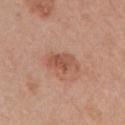Q: Was a biopsy performed?
A: catalogued during a skin exam; not biopsied
Q: Illumination type?
A: white-light illumination
Q: What did automated image analysis measure?
A: a lesion area of about 8 mm², an outline eccentricity of about 0.8 (0 = round, 1 = elongated), and a symmetry-axis asymmetry near 0.25; roughly 9 lightness units darker than nearby skin and a normalized border contrast of about 6.5; internal color variation of about 3.5 on a 0–10 scale and peripheral color asymmetry of about 1
Q: Where on the body is the lesion?
A: the left upper arm
Q: Patient demographics?
A: female, aged 53 to 57
Q: How was this image acquired?
A: ~15 mm crop, total-body skin-cancer survey